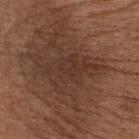Clinical impression:
The lesion was tiled from a total-body skin photograph and was not biopsied.
Clinical summary:
A male patient aged 68 to 72. The lesion is on the chest. The lesion's longest dimension is about 7 mm. This is a white-light tile. The total-body-photography lesion software estimated an average lesion color of about L≈34 a*≈19 b*≈25 (CIELAB) and a normalized border contrast of about 6. The software also gave an automated nevus-likeness rating near 20 out of 100. A region of skin cropped from a whole-body photographic capture, roughly 15 mm wide.